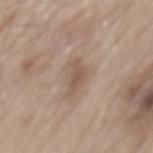Q: Was this lesion biopsied?
A: catalogued during a skin exam; not biopsied
Q: What is the lesion's diameter?
A: about 4 mm
Q: What lighting was used for the tile?
A: white-light
Q: What kind of image is this?
A: ~15 mm tile from a whole-body skin photo
Q: Patient demographics?
A: male, aged 78–82
Q: Lesion location?
A: the mid back